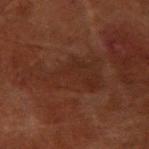This lesion was catalogued during total-body skin photography and was not selected for biopsy.
A female subject, aged 78 to 82.
This is a cross-polarized tile.
Automated tile analysis of the lesion measured a shape eccentricity near 0.85.
Measured at roughly 7 mm in maximum diameter.
From the left forearm.
A 15 mm close-up extracted from a 3D total-body photography capture.